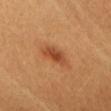biopsy_status: not biopsied; imaged during a skin examination
lesion_size:
  long_diameter_mm_approx: 4.5
site: head or neck
patient:
  sex: female
  age_approx: 30
lighting: cross-polarized
image:
  source: total-body photography crop
  field_of_view_mm: 15
automated_metrics:
  area_mm2_approx: 7.0
  shape_asymmetry: 0.3